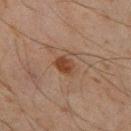Q: Was this lesion biopsied?
A: total-body-photography surveillance lesion; no biopsy
Q: What did automated image analysis measure?
A: a footprint of about 5 mm² and a symmetry-axis asymmetry near 0.2; a peripheral color-asymmetry measure near 1; an automated nevus-likeness rating near 95 out of 100 and a detector confidence of about 100 out of 100 that the crop contains a lesion
Q: Illumination type?
A: cross-polarized illumination
Q: Lesion location?
A: the left thigh
Q: Lesion size?
A: ~2.5 mm (longest diameter)
Q: What kind of image is this?
A: 15 mm crop, total-body photography
Q: What are the patient's age and sex?
A: male, about 45 years old